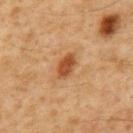Impression:
Recorded during total-body skin imaging; not selected for excision or biopsy.
Acquisition and patient details:
On the mid back. Longest diameter approximately 3.5 mm. A male patient roughly 60 years of age. An algorithmic analysis of the crop reported a footprint of about 5.5 mm². The software also gave a lesion color around L≈46 a*≈24 b*≈37 in CIELAB, roughly 12 lightness units darker than nearby skin, and a normalized border contrast of about 9. The software also gave peripheral color asymmetry of about 0.5. The analysis additionally found a nevus-likeness score of about 95/100 and lesion-presence confidence of about 100/100. Imaged with cross-polarized lighting. Cropped from a total-body skin-imaging series; the visible field is about 15 mm.